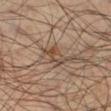{"lesion_size": {"long_diameter_mm_approx": 3.5}, "patient": {"sex": "male", "age_approx": 35}, "site": "right lower leg", "lighting": "cross-polarized", "image": {"source": "total-body photography crop", "field_of_view_mm": 15}, "automated_metrics": {"area_mm2_approx": 5.0, "eccentricity": 0.9, "shape_asymmetry": 0.4, "color_variation_0_10": 6.5, "peripheral_color_asymmetry": 1.5}}